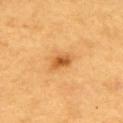Recorded during total-body skin imaging; not selected for excision or biopsy. A close-up tile cropped from a whole-body skin photograph, about 15 mm across. The patient is a male aged around 65. The lesion is on the upper back.